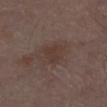Q: Was a biopsy performed?
A: total-body-photography surveillance lesion; no biopsy
Q: How large is the lesion?
A: about 3 mm
Q: Who is the patient?
A: male, aged 68–72
Q: What kind of image is this?
A: 15 mm crop, total-body photography
Q: Lesion location?
A: the left lower leg
Q: What lighting was used for the tile?
A: white-light